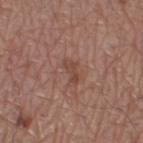Clinical impression: The lesion was photographed on a routine skin check and not biopsied; there is no pathology result. Acquisition and patient details: Cropped from a whole-body photographic skin survey; the tile spans about 15 mm. From the right thigh. The lesion's longest dimension is about 3 mm. The subject is a male roughly 60 years of age. This is a white-light tile.